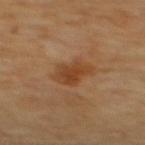Imaged during a routine full-body skin examination; the lesion was not biopsied and no histopathology is available.
The lesion is on the upper back.
About 4 mm across.
A region of skin cropped from a whole-body photographic capture, roughly 15 mm wide.
The subject is aged approximately 70.
Imaged with cross-polarized lighting.